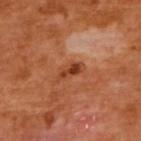subject = female, approximately 55 years of age
tile lighting = cross-polarized
imaging modality = ~15 mm tile from a whole-body skin photo
diameter = ≈3.5 mm
TBP lesion metrics = a lesion color around L≈43 a*≈29 b*≈37 in CIELAB, a lesion–skin lightness drop of about 11, and a normalized border contrast of about 8.5; a classifier nevus-likeness of about 65/100 and a detector confidence of about 100 out of 100 that the crop contains a lesion
site = the upper back